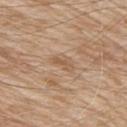Recorded during total-body skin imaging; not selected for excision or biopsy. From the right upper arm. A lesion tile, about 15 mm wide, cut from a 3D total-body photograph. A male patient about 80 years old. An algorithmic analysis of the crop reported an area of roughly 2.5 mm² and two-axis asymmetry of about 0.4. It also reported a mean CIELAB color near L≈56 a*≈17 b*≈33 and a normalized lesion–skin contrast near 5.5. Imaged with white-light lighting.